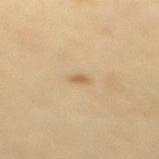– biopsy status · catalogued during a skin exam; not biopsied
– image source · ~15 mm crop, total-body skin-cancer survey
– automated lesion analysis · a nevus-likeness score of about 65/100 and lesion-presence confidence of about 100/100
– diameter · ~1.5 mm (longest diameter)
– site · the mid back
– patient · female, in their mid-60s
– lighting · cross-polarized